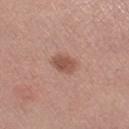Case summary:
- tile lighting: white-light illumination
- patient: female, in their mid- to late 60s
- site: the left thigh
- imaging modality: 15 mm crop, total-body photography
- size: ≈3 mm